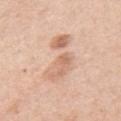Assessment:
No biopsy was performed on this lesion — it was imaged during a full skin examination and was not determined to be concerning.
Clinical summary:
The lesion-visualizer software estimated a lesion area of about 17 mm², an outline eccentricity of about 0.9 (0 = round, 1 = elongated), and two-axis asymmetry of about 0.45. The software also gave a detector confidence of about 100 out of 100 that the crop contains a lesion. The subject is a male aged 48 to 52. This is a white-light tile. The lesion is on the arm. Approximately 6.5 mm at its widest. A lesion tile, about 15 mm wide, cut from a 3D total-body photograph.